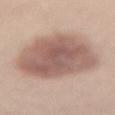notes — total-body-photography surveillance lesion; no biopsy
body site — the lower back
subject — female, aged around 55
tile lighting — white-light
size — ≈10 mm
image source — 15 mm crop, total-body photography